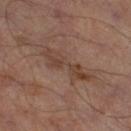{
  "biopsy_status": "not biopsied; imaged during a skin examination",
  "image": {
    "source": "total-body photography crop",
    "field_of_view_mm": 15
  },
  "patient": {
    "sex": "male",
    "age_approx": 65
  },
  "site": "leg",
  "lesion_size": {
    "long_diameter_mm_approx": 6.5
  }
}